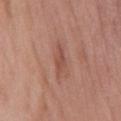Clinical impression:
Captured during whole-body skin photography for melanoma surveillance; the lesion was not biopsied.
Acquisition and patient details:
The recorded lesion diameter is about 3 mm. The tile uses white-light illumination. A male subject aged around 55. A region of skin cropped from a whole-body photographic capture, roughly 15 mm wide. Located on the chest.